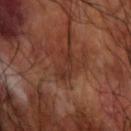Clinical impression:
The lesion was tiled from a total-body skin photograph and was not biopsied.
Context:
Imaged with cross-polarized lighting. A male subject approximately 60 years of age. Longest diameter approximately 4 mm. A 15 mm close-up tile from a total-body photography series done for melanoma screening. From the right forearm. The total-body-photography lesion software estimated a mean CIELAB color near L≈32 a*≈22 b*≈26 and a normalized border contrast of about 6. The software also gave a detector confidence of about 75 out of 100 that the crop contains a lesion.